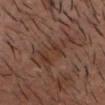Assessment: This lesion was catalogued during total-body skin photography and was not selected for biopsy. Context: A lesion tile, about 15 mm wide, cut from a 3D total-body photograph. A male patient in their 40s. The lesion is on the head or neck. About 6 mm across. The tile uses cross-polarized illumination.